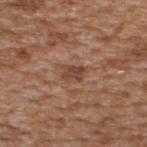Captured under white-light illumination.
Approximately 2.5 mm at its widest.
A 15 mm close-up extracted from a 3D total-body photography capture.
A male subject, aged 63 to 67.
Located on the upper back.